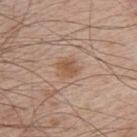Case summary:
• biopsy status · no biopsy performed (imaged during a skin exam)
• image source · ~15 mm tile from a whole-body skin photo
• automated metrics · a footprint of about 4.5 mm² and an outline eccentricity of about 0.55 (0 = round, 1 = elongated); a lesion–skin lightness drop of about 8 and a normalized border contrast of about 7; a border-irregularity index near 2/10, a color-variation rating of about 2/10, and radial color variation of about 0.5
• illumination · white-light
• location · the arm
• lesion diameter · ~2.5 mm (longest diameter)
• patient · male, approximately 65 years of age